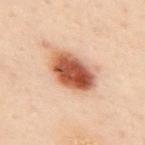notes = imaged on a skin check; not biopsied
site = the upper back
subject = male, aged 48 to 52
imaging modality = total-body-photography crop, ~15 mm field of view